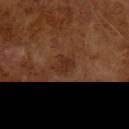No biopsy was performed on this lesion — it was imaged during a full skin examination and was not determined to be concerning. Imaged with cross-polarized lighting. Cropped from a total-body skin-imaging series; the visible field is about 15 mm. A male subject about 65 years old. The total-body-photography lesion software estimated an area of roughly 4 mm², a shape eccentricity near 0.7, and two-axis asymmetry of about 0.3. And it measured a lesion–skin lightness drop of about 6 and a lesion-to-skin contrast of about 6 (normalized; higher = more distinct). Longest diameter approximately 2.5 mm.